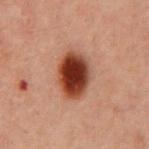Q: Is there a histopathology result?
A: total-body-photography surveillance lesion; no biopsy
Q: What kind of image is this?
A: ~15 mm tile from a whole-body skin photo
Q: What is the anatomic site?
A: the chest
Q: Who is the patient?
A: male, roughly 60 years of age